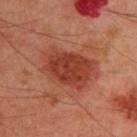{
  "biopsy_status": "not biopsied; imaged during a skin examination",
  "site": "upper back",
  "automated_metrics": {
    "cielab_L": 41,
    "cielab_a": 31,
    "cielab_b": 32,
    "vs_skin_contrast_norm": 8.5,
    "border_irregularity_0_10": 2.0,
    "color_variation_0_10": 3.5,
    "peripheral_color_asymmetry": 1.0,
    "nevus_likeness_0_100": 100
  },
  "image": {
    "source": "total-body photography crop",
    "field_of_view_mm": 15
  },
  "patient": {
    "sex": "male",
    "age_approx": 50
  },
  "lighting": "cross-polarized"
}